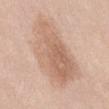biopsy status = total-body-photography surveillance lesion; no biopsy | location = the right thigh | subject = female, roughly 20 years of age | lighting = white-light illumination | automated metrics = an area of roughly 38 mm², an eccentricity of roughly 0.8, and a symmetry-axis asymmetry near 0.25; a mean CIELAB color near L≈64 a*≈18 b*≈29 and about 9 CIELAB-L* units darker than the surrounding skin; a border-irregularity index near 3/10, internal color variation of about 5 on a 0–10 scale, and peripheral color asymmetry of about 1.5 | size = about 9.5 mm | acquisition = ~15 mm tile from a whole-body skin photo.